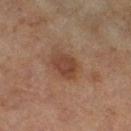| key | value |
|---|---|
| patient | female, aged 58–62 |
| diameter | about 3.5 mm |
| site | the right thigh |
| imaging modality | ~15 mm tile from a whole-body skin photo |